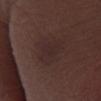The lesion-visualizer software estimated a lesion color around L≈25 a*≈15 b*≈16 in CIELAB and a lesion-to-skin contrast of about 5.5 (normalized; higher = more distinct). The analysis additionally found a nevus-likeness score of about 0/100 and a detector confidence of about 100 out of 100 that the crop contains a lesion. A lesion tile, about 15 mm wide, cut from a 3D total-body photograph. The lesion is located on the leg. The tile uses white-light illumination. A male patient, aged 68 to 72. About 5 mm across.